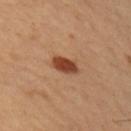Imaged during a routine full-body skin examination; the lesion was not biopsied and no histopathology is available. Captured under cross-polarized illumination. Longest diameter approximately 3 mm. On the right upper arm. A lesion tile, about 15 mm wide, cut from a 3D total-body photograph. A male patient, in their mid-50s.